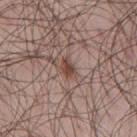Part of a total-body skin-imaging series; this lesion was reviewed on a skin check and was not flagged for biopsy.
A male subject aged around 45.
About 2.5 mm across.
This image is a 15 mm lesion crop taken from a total-body photograph.
On the mid back.
Captured under white-light illumination.